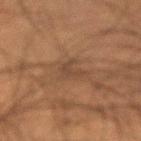biopsy status: total-body-photography surveillance lesion; no biopsy | patient: male, roughly 50 years of age | location: the left upper arm | lesion size: ~2.5 mm (longest diameter) | image: ~15 mm tile from a whole-body skin photo | lighting: cross-polarized.